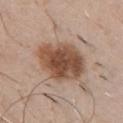Recorded during total-body skin imaging; not selected for excision or biopsy. The tile uses white-light illumination. From the chest. The lesion-visualizer software estimated an average lesion color of about L≈50 a*≈19 b*≈29 (CIELAB). The software also gave a classifier nevus-likeness of about 95/100 and lesion-presence confidence of about 100/100. A 15 mm close-up extracted from a 3D total-body photography capture. A male patient aged around 50. Measured at roughly 6.5 mm in maximum diameter.